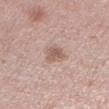Clinical impression:
The lesion was photographed on a routine skin check and not biopsied; there is no pathology result.
Acquisition and patient details:
On the left lower leg. The lesion's longest dimension is about 2.5 mm. A region of skin cropped from a whole-body photographic capture, roughly 15 mm wide. A female subject in their 40s.